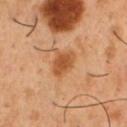follow-up = total-body-photography surveillance lesion; no biopsy
acquisition = ~15 mm crop, total-body skin-cancer survey
subject = male, in their 50s
illumination = cross-polarized
lesion diameter = about 3.5 mm
site = the chest